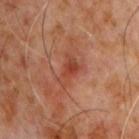The lesion was tiled from a total-body skin photograph and was not biopsied. Located on the chest. About 3.5 mm across. Cropped from a whole-body photographic skin survey; the tile spans about 15 mm. Automated image analysis of the tile measured an area of roughly 5.5 mm², an outline eccentricity of about 0.8 (0 = round, 1 = elongated), and a shape-asymmetry score of about 0.5 (0 = symmetric). The analysis additionally found a border-irregularity rating of about 5.5/10, a color-variation rating of about 3/10, and a peripheral color-asymmetry measure near 1. A male subject, about 60 years old.